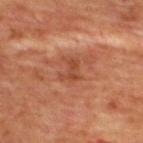  biopsy_status: not biopsied; imaged during a skin examination
  image:
    source: total-body photography crop
    field_of_view_mm: 15
  patient:
    sex: female
    age_approx: 45
  automated_metrics:
    border_irregularity_0_10: 5.5
    color_variation_0_10: 1.5
    peripheral_color_asymmetry: 0.0
  lesion_size:
    long_diameter_mm_approx: 3.0
  site: back
  lighting: cross-polarized